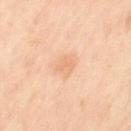Assessment: Captured during whole-body skin photography for melanoma surveillance; the lesion was not biopsied. Clinical summary: The tile uses cross-polarized illumination. The patient is a female aged approximately 50. A 15 mm close-up extracted from a 3D total-body photography capture. Approximately 2.5 mm at its widest. From the left thigh. Automated image analysis of the tile measured a footprint of about 4.5 mm².